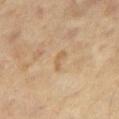notes — no biopsy performed (imaged during a skin exam)
lesion size — about 2.5 mm
subject — male, aged 63 to 67
lighting — cross-polarized illumination
acquisition — 15 mm crop, total-body photography